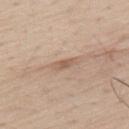Recorded during total-body skin imaging; not selected for excision or biopsy. From the upper back. Imaged with white-light lighting. A close-up tile cropped from a whole-body skin photograph, about 15 mm across. A male patient, in their mid- to late 70s. About 3 mm across.